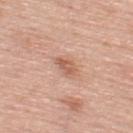follow-up: total-body-photography surveillance lesion; no biopsy | automated lesion analysis: a mean CIELAB color near L≈59 a*≈23 b*≈31, about 10 CIELAB-L* units darker than the surrounding skin, and a lesion-to-skin contrast of about 6.5 (normalized; higher = more distinct); border irregularity of about 2 on a 0–10 scale, internal color variation of about 3 on a 0–10 scale, and radial color variation of about 1; a nevus-likeness score of about 5/100 and a detector confidence of about 100 out of 100 that the crop contains a lesion | lesion size: about 2.5 mm | subject: male, in their mid-50s | illumination: white-light | body site: the upper back | image source: total-body-photography crop, ~15 mm field of view.